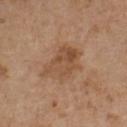A male subject aged around 70.
This is a white-light tile.
The recorded lesion diameter is about 6 mm.
The lesion is located on the front of the torso.
A close-up tile cropped from a whole-body skin photograph, about 15 mm across.
Automated image analysis of the tile measured a lesion color around L≈50 a*≈19 b*≈33 in CIELAB, about 8 CIELAB-L* units darker than the surrounding skin, and a lesion-to-skin contrast of about 6.5 (normalized; higher = more distinct). The software also gave an automated nevus-likeness rating near 5 out of 100.